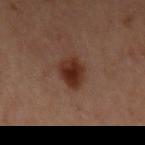| key | value |
|---|---|
| follow-up | catalogued during a skin exam; not biopsied |
| lighting | cross-polarized |
| subject | female, in their mid-40s |
| imaging modality | ~15 mm crop, total-body skin-cancer survey |
| TBP lesion metrics | an area of roughly 8 mm², an outline eccentricity of about 0.5 (0 = round, 1 = elongated), and two-axis asymmetry of about 0.25; a lesion color around L≈29 a*≈21 b*≈25 in CIELAB, roughly 11 lightness units darker than nearby skin, and a normalized border contrast of about 11; border irregularity of about 2.5 on a 0–10 scale and a peripheral color-asymmetry measure near 1; lesion-presence confidence of about 100/100 |
| location | the left upper arm |